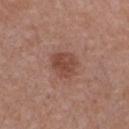biopsy status: total-body-photography surveillance lesion; no biopsy
image: total-body-photography crop, ~15 mm field of view
patient: female, aged 38 to 42
tile lighting: white-light illumination
body site: the chest
lesion size: ≈3 mm
automated lesion analysis: a shape-asymmetry score of about 0.2 (0 = symmetric); a lesion–skin lightness drop of about 9; a color-variation rating of about 3/10 and peripheral color asymmetry of about 1; a classifier nevus-likeness of about 35/100 and a detector confidence of about 100 out of 100 that the crop contains a lesion